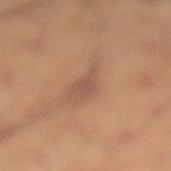Case summary:
– biopsy status: catalogued during a skin exam; not biopsied
– tile lighting: cross-polarized illumination
– body site: the leg
– imaging modality: ~15 mm crop, total-body skin-cancer survey
– patient: male, roughly 55 years of age
– lesion size: ~3.5 mm (longest diameter)